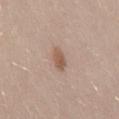This lesion was catalogued during total-body skin photography and was not selected for biopsy. A 15 mm crop from a total-body photograph taken for skin-cancer surveillance. The lesion is located on the mid back. The patient is a male in their 30s. Measured at roughly 2.5 mm in maximum diameter. Captured under white-light illumination.